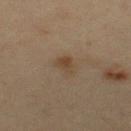The lesion was photographed on a routine skin check and not biopsied; there is no pathology result.
A 15 mm close-up extracted from a 3D total-body photography capture.
Automated image analysis of the tile measured a lesion color around L≈41 a*≈13 b*≈29 in CIELAB, roughly 6 lightness units darker than nearby skin, and a normalized lesion–skin contrast near 6.5. It also reported a classifier nevus-likeness of about 30/100 and lesion-presence confidence of about 100/100.
A male patient aged 33 to 37.
This is a cross-polarized tile.
The lesion is on the upper back.
About 2.5 mm across.